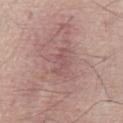biopsy status: total-body-photography surveillance lesion; no biopsy | subject: male, aged approximately 65 | imaging modality: ~15 mm crop, total-body skin-cancer survey | anatomic site: the abdomen.